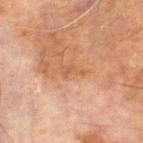Imaged during a routine full-body skin examination; the lesion was not biopsied and no histopathology is available.
An algorithmic analysis of the crop reported a lesion area of about 2.5 mm² and two-axis asymmetry of about 0.45.
A close-up tile cropped from a whole-body skin photograph, about 15 mm across.
The lesion is on the left lower leg.
The tile uses cross-polarized illumination.
A male patient aged 68–72.